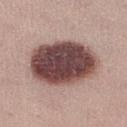Clinical impression:
The lesion was photographed on a routine skin check and not biopsied; there is no pathology result.
Image and clinical context:
A close-up tile cropped from a whole-body skin photograph, about 15 mm across. Located on the right lower leg. The subject is a female aged approximately 25. Measured at roughly 8 mm in maximum diameter.